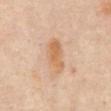Captured during whole-body skin photography for melanoma surveillance; the lesion was not biopsied.
The tile uses white-light illumination.
Cropped from a total-body skin-imaging series; the visible field is about 15 mm.
The patient is a female approximately 65 years of age.
From the abdomen.
The lesion's longest dimension is about 4.5 mm.